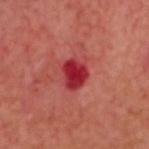  biopsy_status: not biopsied; imaged during a skin examination
  site: head or neck
  patient:
    sex: male
    age_approx: 50
  lesion_size:
    long_diameter_mm_approx: 3.5
  image:
    source: total-body photography crop
    field_of_view_mm: 15
  automated_metrics:
    area_mm2_approx: 8.0
    eccentricity: 0.55
    shape_asymmetry: 0.2
    border_irregularity_0_10: 1.5
  lighting: cross-polarized